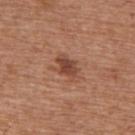Notes:
- notes: catalogued during a skin exam; not biopsied
- image source: ~15 mm tile from a whole-body skin photo
- illumination: white-light
- lesion size: ≈3.5 mm
- location: the upper back
- subject: male, about 65 years old